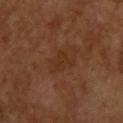biopsy status: imaged on a skin check; not biopsied
TBP lesion metrics: a lesion area of about 4 mm², an outline eccentricity of about 0.75 (0 = round, 1 = elongated), and a symmetry-axis asymmetry near 0.3; an automated nevus-likeness rating near 0 out of 100 and a lesion-detection confidence of about 100/100
lesion diameter: ~2.5 mm (longest diameter)
location: the upper back
illumination: cross-polarized illumination
subject: male, aged 63–67
acquisition: 15 mm crop, total-body photography